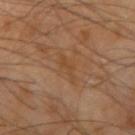Case summary:
- notes · catalogued during a skin exam; not biopsied
- body site · the right upper arm
- automated metrics · a lesion color around L≈45 a*≈20 b*≈35 in CIELAB and roughly 5 lightness units darker than nearby skin; a border-irregularity rating of about 7/10 and a within-lesion color-variation index near 0/10; a classifier nevus-likeness of about 0/100 and lesion-presence confidence of about 100/100
- tile lighting · cross-polarized
- image source · total-body-photography crop, ~15 mm field of view
- subject · male, aged 63–67
- size · about 3 mm